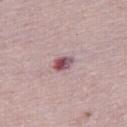notes — catalogued during a skin exam; not biopsied
patient — female, aged 53 to 57
body site — the leg
image — 15 mm crop, total-body photography